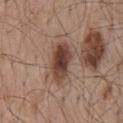Q: Was this lesion biopsied?
A: imaged on a skin check; not biopsied
Q: Where on the body is the lesion?
A: the back
Q: Who is the patient?
A: male, approximately 55 years of age
Q: What kind of image is this?
A: 15 mm crop, total-body photography
Q: Automated lesion metrics?
A: a lesion area of about 11 mm², an outline eccentricity of about 0.85 (0 = round, 1 = elongated), and a shape-asymmetry score of about 0.15 (0 = symmetric); roughly 14 lightness units darker than nearby skin and a normalized border contrast of about 10.5; a border-irregularity rating of about 2/10, a color-variation rating of about 6/10, and a peripheral color-asymmetry measure near 1.5; an automated nevus-likeness rating near 100 out of 100 and lesion-presence confidence of about 100/100
Q: What is the lesion's diameter?
A: ~5 mm (longest diameter)